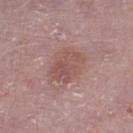Background:
A 15 mm close-up tile from a total-body photography series done for melanoma screening. Approximately 4 mm at its widest. A male patient approximately 75 years of age. An algorithmic analysis of the crop reported an average lesion color of about L≈52 a*≈22 b*≈22 (CIELAB), roughly 8 lightness units darker than nearby skin, and a normalized lesion–skin contrast near 6. It also reported a color-variation rating of about 3.5/10. This is a white-light tile. On the left lower leg.